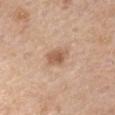Clinical impression: Recorded during total-body skin imaging; not selected for excision or biopsy. Image and clinical context: Located on the arm. A male patient, in their mid-70s. A 15 mm crop from a total-body photograph taken for skin-cancer surveillance. The tile uses white-light illumination. The lesion's longest dimension is about 2.5 mm.